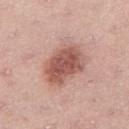Image and clinical context:
A region of skin cropped from a whole-body photographic capture, roughly 15 mm wide. The lesion is on the right thigh. The subject is a female in their 40s.